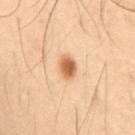Q: Is there a histopathology result?
A: imaged on a skin check; not biopsied
Q: Who is the patient?
A: male, roughly 50 years of age
Q: Automated lesion metrics?
A: a lesion area of about 4.5 mm², an eccentricity of roughly 0.65, and two-axis asymmetry of about 0.2; a lesion color around L≈47 a*≈18 b*≈32 in CIELAB and a lesion–skin lightness drop of about 12; a border-irregularity index near 1.5/10; a nevus-likeness score of about 100/100 and a lesion-detection confidence of about 100/100
Q: What is the imaging modality?
A: ~15 mm tile from a whole-body skin photo
Q: Lesion size?
A: ~2.5 mm (longest diameter)
Q: Lesion location?
A: the abdomen